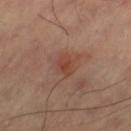Q: Was a biopsy performed?
A: total-body-photography surveillance lesion; no biopsy
Q: How was this image acquired?
A: ~15 mm tile from a whole-body skin photo
Q: How large is the lesion?
A: ≈2.5 mm
Q: What is the anatomic site?
A: the left thigh